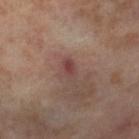Captured during whole-body skin photography for melanoma surveillance; the lesion was not biopsied. Located on the right thigh. The tile uses cross-polarized illumination. This image is a 15 mm lesion crop taken from a total-body photograph. The subject is a female aged 53–57. Automated image analysis of the tile measured an area of roughly 3.5 mm² and a shape eccentricity near 0.85. And it measured internal color variation of about 2 on a 0–10 scale and a peripheral color-asymmetry measure near 0.5.